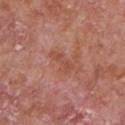| field | value |
|---|---|
| follow-up | no biopsy performed (imaged during a skin exam) |
| anatomic site | the chest |
| imaging modality | ~15 mm tile from a whole-body skin photo |
| subject | male, aged 63–67 |